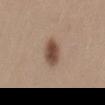<lesion>
<image>
  <source>total-body photography crop</source>
  <field_of_view_mm>15</field_of_view_mm>
</image>
<site>lower back</site>
<patient>
  <sex>female</sex>
  <age_approx>40</age_approx>
</patient>
</lesion>